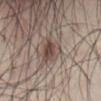Part of a total-body skin-imaging series; this lesion was reviewed on a skin check and was not flagged for biopsy.
The recorded lesion diameter is about 3.5 mm.
On the abdomen.
A 15 mm close-up extracted from a 3D total-body photography capture.
This is a white-light tile.
The patient is a male aged 23 to 27.
The lesion-visualizer software estimated border irregularity of about 3 on a 0–10 scale, a color-variation rating of about 6.5/10, and peripheral color asymmetry of about 2.5. The software also gave an automated nevus-likeness rating near 90 out of 100.